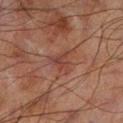| field | value |
|---|---|
| notes | catalogued during a skin exam; not biopsied |
| illumination | cross-polarized illumination |
| automated lesion analysis | an average lesion color of about L≈34 a*≈20 b*≈22 (CIELAB); a border-irregularity index near 6/10, a color-variation rating of about 3/10, and peripheral color asymmetry of about 1; an automated nevus-likeness rating near 0 out of 100 and a detector confidence of about 100 out of 100 that the crop contains a lesion |
| location | the left lower leg |
| diameter | about 3 mm |
| image | 15 mm crop, total-body photography |
| subject | male, aged 68–72 |